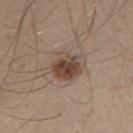<tbp_lesion>
  <biopsy_status>not biopsied; imaged during a skin examination</biopsy_status>
  <lighting>white-light</lighting>
  <image>
    <source>total-body photography crop</source>
    <field_of_view_mm>15</field_of_view_mm>
  </image>
  <site>right upper arm</site>
  <patient>
    <sex>male</sex>
    <age_approx>30</age_approx>
  </patient>
  <lesion_size>
    <long_diameter_mm_approx>4.0</long_diameter_mm_approx>
  </lesion_size>
</tbp_lesion>